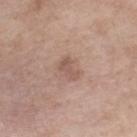Captured during whole-body skin photography for melanoma surveillance; the lesion was not biopsied. The lesion's longest dimension is about 3 mm. The patient is a female approximately 55 years of age. An algorithmic analysis of the crop reported a footprint of about 3 mm², a shape eccentricity near 0.9, and a symmetry-axis asymmetry near 0.45. The software also gave an average lesion color of about L≈54 a*≈19 b*≈25 (CIELAB). The analysis additionally found a border-irregularity rating of about 5/10, a color-variation rating of about 0/10, and peripheral color asymmetry of about 0. A lesion tile, about 15 mm wide, cut from a 3D total-body photograph. Imaged with white-light lighting. The lesion is on the right lower leg.